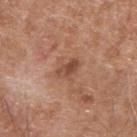  biopsy_status: not biopsied; imaged during a skin examination
  image:
    source: total-body photography crop
    field_of_view_mm: 15
  lesion_size:
    long_diameter_mm_approx: 3.0
  automated_metrics:
    cielab_L: 48
    cielab_a: 23
    cielab_b: 30
    vs_skin_contrast_norm: 7.5
    nevus_likeness_0_100: 5
    lesion_detection_confidence_0_100: 100
  patient:
    sex: male
    age_approx: 75
  site: arm